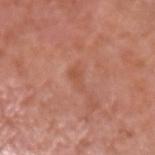workup = imaged on a skin check; not biopsied